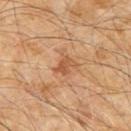Impression:
The lesion was tiled from a total-body skin photograph and was not biopsied.
Background:
The subject is a male aged approximately 60. Cropped from a total-body skin-imaging series; the visible field is about 15 mm. The lesion is on the chest. The lesion's longest dimension is about 2.5 mm.